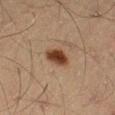No biopsy was performed on this lesion — it was imaged during a full skin examination and was not determined to be concerning. This image is a 15 mm lesion crop taken from a total-body photograph. A male patient approximately 45 years of age. About 3 mm across. Located on the left thigh. Automated image analysis of the tile measured a lesion area of about 6 mm², an eccentricity of roughly 0.65, and two-axis asymmetry of about 0.25.